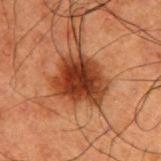Clinical impression: The lesion was photographed on a routine skin check and not biopsied; there is no pathology result. Clinical summary: Captured under cross-polarized illumination. The subject is a male approximately 50 years of age. This image is a 15 mm lesion crop taken from a total-body photograph. On the upper back.